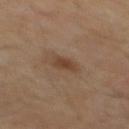<lesion>
<biopsy_status>not biopsied; imaged during a skin examination</biopsy_status>
<lighting>cross-polarized</lighting>
<site>back</site>
<automated_metrics>
  <area_mm2_approx>4.0</area_mm2_approx>
  <eccentricity>0.75</eccentricity>
  <shape_asymmetry>0.25</shape_asymmetry>
  <vs_skin_darker_L>8.0</vs_skin_darker_L>
  <vs_skin_contrast_norm>7.5</vs_skin_contrast_norm>
  <color_variation_0_10>1.5</color_variation_0_10>
  <peripheral_color_asymmetry>0.5</peripheral_color_asymmetry>
  <nevus_likeness_0_100>65</nevus_likeness_0_100>
</automated_metrics>
<patient>
  <sex>male</sex>
  <age_approx>55</age_approx>
</patient>
<lesion_size>
  <long_diameter_mm_approx>3.0</long_diameter_mm_approx>
</lesion_size>
<image>
  <source>total-body photography crop</source>
  <field_of_view_mm>15</field_of_view_mm>
</image>
</lesion>